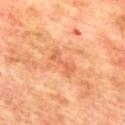The lesion was tiled from a total-body skin photograph and was not biopsied. On the mid back. Captured under cross-polarized illumination. A male subject, aged 73–77. A lesion tile, about 15 mm wide, cut from a 3D total-body photograph.